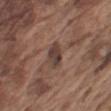The lesion was tiled from a total-body skin photograph and was not biopsied. A 15 mm close-up tile from a total-body photography series done for melanoma screening. Automated image analysis of the tile measured a lesion-to-skin contrast of about 9 (normalized; higher = more distinct). The software also gave a border-irregularity index near 2/10. The subject is a male aged around 75. Longest diameter approximately 3 mm. From the left upper arm. This is a white-light tile.